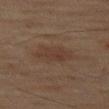notes: no biopsy performed (imaged during a skin exam) | patient: female, aged approximately 60 | anatomic site: the left thigh | image-analysis metrics: an eccentricity of roughly 0.85 and two-axis asymmetry of about 0.25; a mean CIELAB color near L≈33 a*≈15 b*≈23, roughly 5 lightness units darker than nearby skin, and a normalized border contrast of about 5.5; a border-irregularity index near 3/10, a color-variation rating of about 2.5/10, and peripheral color asymmetry of about 1; a classifier nevus-likeness of about 15/100 and lesion-presence confidence of about 100/100 | lesion diameter: about 5 mm | lighting: cross-polarized | image source: total-body-photography crop, ~15 mm field of view.